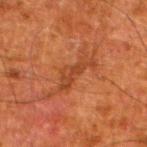<record>
<biopsy_status>not biopsied; imaged during a skin examination</biopsy_status>
<lesion_size>
  <long_diameter_mm_approx>4.5</long_diameter_mm_approx>
</lesion_size>
<site>left lower leg</site>
<patient>
  <sex>male</sex>
  <age_approx>80</age_approx>
</patient>
<image>
  <source>total-body photography crop</source>
  <field_of_view_mm>15</field_of_view_mm>
</image>
<lighting>cross-polarized</lighting>
</record>